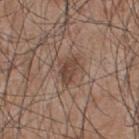Q: Was a biopsy performed?
A: total-body-photography surveillance lesion; no biopsy
Q: What lighting was used for the tile?
A: white-light illumination
Q: What kind of image is this?
A: 15 mm crop, total-body photography
Q: What is the anatomic site?
A: the back
Q: Patient demographics?
A: male, in their mid- to late 40s